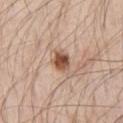Assessment:
The lesion was tiled from a total-body skin photograph and was not biopsied.
Context:
The lesion is on the right upper arm. A roughly 15 mm field-of-view crop from a total-body skin photograph. The recorded lesion diameter is about 2.5 mm. This is a white-light tile. A male patient approximately 70 years of age. Automated tile analysis of the lesion measured a lesion area of about 4.5 mm², an outline eccentricity of about 0.55 (0 = round, 1 = elongated), and a symmetry-axis asymmetry near 0.2. The analysis additionally found a lesion color around L≈52 a*≈21 b*≈30 in CIELAB, roughly 16 lightness units darker than nearby skin, and a normalized border contrast of about 11. It also reported a nevus-likeness score of about 95/100 and a detector confidence of about 100 out of 100 that the crop contains a lesion.